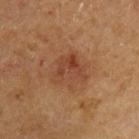notes: no biopsy performed (imaged during a skin exam)
acquisition: total-body-photography crop, ~15 mm field of view
patient: male, aged 63 to 67
TBP lesion metrics: a footprint of about 9 mm², an outline eccentricity of about 0.5 (0 = round, 1 = elongated), and two-axis asymmetry of about 0.45; border irregularity of about 4.5 on a 0–10 scale and a peripheral color-asymmetry measure near 1.5; a nevus-likeness score of about 5/100
anatomic site: the chest
diameter: ≈4 mm
lighting: cross-polarized